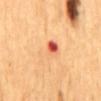Recorded during total-body skin imaging; not selected for excision or biopsy.
A male patient aged approximately 65.
Measured at roughly 4 mm in maximum diameter.
The lesion is on the back.
Imaged with cross-polarized lighting.
Cropped from a whole-body photographic skin survey; the tile spans about 15 mm.